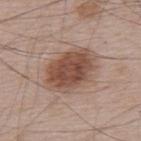{
  "biopsy_status": "not biopsied; imaged during a skin examination",
  "image": {
    "source": "total-body photography crop",
    "field_of_view_mm": 15
  },
  "site": "upper back",
  "patient": {
    "sex": "male",
    "age_approx": 65
  },
  "automated_metrics": {
    "vs_skin_darker_L": 13.0,
    "vs_skin_contrast_norm": 9.5,
    "color_variation_0_10": 4.5,
    "peripheral_color_asymmetry": 1.5,
    "nevus_likeness_0_100": 95,
    "lesion_detection_confidence_0_100": 100
  },
  "lighting": "white-light"
}